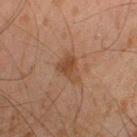| key | value |
|---|---|
| notes | no biopsy performed (imaged during a skin exam) |
| automated lesion analysis | an average lesion color of about L≈36 a*≈17 b*≈27 (CIELAB), a lesion–skin lightness drop of about 7, and a normalized border contrast of about 7 |
| lighting | cross-polarized |
| subject | male, in their mid-40s |
| site | the right lower leg |
| imaging modality | 15 mm crop, total-body photography |
| diameter | about 3.5 mm |